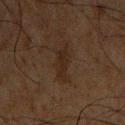biopsy status: imaged on a skin check; not biopsied
tile lighting: cross-polarized illumination
image: ~15 mm tile from a whole-body skin photo
subject: male, about 60 years old
body site: the chest